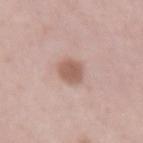* follow-up: imaged on a skin check; not biopsied
* anatomic site: the abdomen
* image: ~15 mm tile from a whole-body skin photo
* patient: male, aged approximately 60